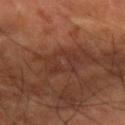| key | value |
|---|---|
| subject | male, approximately 55 years of age |
| body site | the right forearm |
| illumination | cross-polarized illumination |
| acquisition | 15 mm crop, total-body photography |